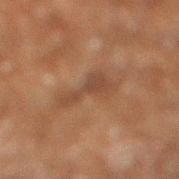A male patient aged 58 to 62.
Measured at roughly 5 mm in maximum diameter.
Cropped from a total-body skin-imaging series; the visible field is about 15 mm.
Located on the right lower leg.
Imaged with cross-polarized lighting.